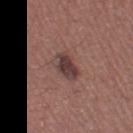Notes:
– follow-up — catalogued during a skin exam; not biopsied
– acquisition — total-body-photography crop, ~15 mm field of view
– lesion diameter — ≈3.5 mm
– site — the left thigh
– subject — male, aged 43 to 47
– tile lighting — white-light
– automated lesion analysis — a lesion area of about 7 mm² and an eccentricity of roughly 0.7; radial color variation of about 1.5; a classifier nevus-likeness of about 40/100 and a detector confidence of about 100 out of 100 that the crop contains a lesion